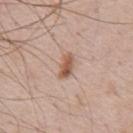| key | value |
|---|---|
| notes | total-body-photography surveillance lesion; no biopsy |
| image | total-body-photography crop, ~15 mm field of view |
| automated metrics | an eccentricity of roughly 0.85; a lesion–skin lightness drop of about 11 and a normalized lesion–skin contrast near 8.5; a border-irregularity index near 2/10 and a color-variation rating of about 5/10 |
| site | the chest |
| illumination | white-light illumination |
| subject | male, aged 68 to 72 |